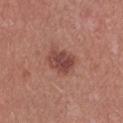Notes:
- notes — catalogued during a skin exam; not biopsied
- patient — female, aged approximately 25
- location — the chest
- acquisition — total-body-photography crop, ~15 mm field of view
- diameter — ~3.5 mm (longest diameter)
- illumination — white-light illumination
- TBP lesion metrics — a normalized lesion–skin contrast near 8.5; a border-irregularity rating of about 2/10, a within-lesion color-variation index near 3.5/10, and a peripheral color-asymmetry measure near 1; a nevus-likeness score of about 55/100 and a lesion-detection confidence of about 100/100